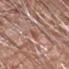| key | value |
|---|---|
| notes | no biopsy performed (imaged during a skin exam) |
| lesion diameter | about 2.5 mm |
| subject | male, aged 68 to 72 |
| anatomic site | the right forearm |
| image-analysis metrics | a footprint of about 2 mm², an outline eccentricity of about 0.95 (0 = round, 1 = elongated), and two-axis asymmetry of about 0.25; an average lesion color of about L≈52 a*≈21 b*≈26 (CIELAB), roughly 8 lightness units darker than nearby skin, and a normalized lesion–skin contrast near 6 |
| image | ~15 mm tile from a whole-body skin photo |
| tile lighting | white-light |